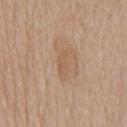biopsy status = catalogued during a skin exam; not biopsied
subject = male, aged 63–67
diameter = about 3.5 mm
site = the front of the torso
tile lighting = white-light illumination
imaging modality = total-body-photography crop, ~15 mm field of view